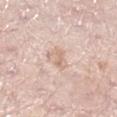Q: Was this lesion biopsied?
A: total-body-photography surveillance lesion; no biopsy
Q: Lesion location?
A: the right lower leg
Q: What is the imaging modality?
A: ~15 mm crop, total-body skin-cancer survey
Q: Patient demographics?
A: female, aged 68 to 72